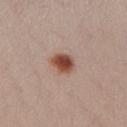Clinical impression: This lesion was catalogued during total-body skin photography and was not selected for biopsy. Context: Captured under white-light illumination. A close-up tile cropped from a whole-body skin photograph, about 15 mm across. A female subject, aged around 25. The recorded lesion diameter is about 3 mm. From the right lower leg.